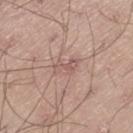Imaged during a routine full-body skin examination; the lesion was not biopsied and no histopathology is available. The subject is a male aged approximately 20. The lesion is on the left thigh. A lesion tile, about 15 mm wide, cut from a 3D total-body photograph. About 3.5 mm across. Captured under white-light illumination.